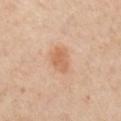biopsy status = no biopsy performed (imaged during a skin exam) | image = ~15 mm tile from a whole-body skin photo | patient = male, in their mid-60s | site = the chest.